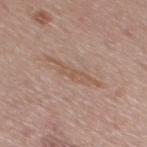| field | value |
|---|---|
| biopsy status | imaged on a skin check; not biopsied |
| automated metrics | about 7 CIELAB-L* units darker than the surrounding skin and a normalized lesion–skin contrast near 5.5 |
| anatomic site | the mid back |
| subject | male, aged 53 to 57 |
| acquisition | 15 mm crop, total-body photography |
| size | about 5.5 mm |